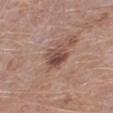The lesion was tiled from a total-body skin photograph and was not biopsied. The lesion is located on the left lower leg. The recorded lesion diameter is about 3.5 mm. A close-up tile cropped from a whole-body skin photograph, about 15 mm across. The patient is a male aged around 50. The total-body-photography lesion software estimated roughly 11 lightness units darker than nearby skin and a normalized lesion–skin contrast near 8. It also reported lesion-presence confidence of about 100/100.